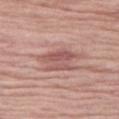{
  "lighting": "white-light",
  "image": {
    "source": "total-body photography crop",
    "field_of_view_mm": 15
  },
  "patient": {
    "sex": "female",
    "age_approx": 70
  },
  "lesion_size": {
    "long_diameter_mm_approx": 5.0
  },
  "site": "leg",
  "automated_metrics": {
    "border_irregularity_0_10": 4.5,
    "peripheral_color_asymmetry": 1.5
  }
}